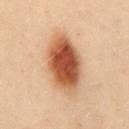notes = imaged on a skin check; not biopsied | patient = female, approximately 30 years of age | site = the mid back | image = ~15 mm tile from a whole-body skin photo.